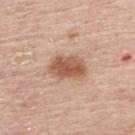Part of a total-body skin-imaging series; this lesion was reviewed on a skin check and was not flagged for biopsy. Measured at roughly 4.5 mm in maximum diameter. A 15 mm close-up extracted from a 3D total-body photography capture. Located on the back. Captured under white-light illumination. A male subject aged 53 to 57.